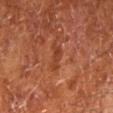No biopsy was performed on this lesion — it was imaged during a full skin examination and was not determined to be concerning. A region of skin cropped from a whole-body photographic capture, roughly 15 mm wide. On the left lower leg. Captured under cross-polarized illumination. A male subject, about 65 years old. The recorded lesion diameter is about 3 mm.